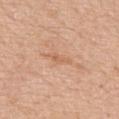{"biopsy_status": "not biopsied; imaged during a skin examination", "patient": {"sex": "male", "age_approx": 75}, "lesion_size": {"long_diameter_mm_approx": 2.5}, "lighting": "white-light", "image": {"source": "total-body photography crop", "field_of_view_mm": 15}, "site": "upper back", "automated_metrics": {"cielab_L": 62, "cielab_a": 22, "cielab_b": 36, "vs_skin_darker_L": 7.0, "vs_skin_contrast_norm": 5.0, "color_variation_0_10": 0.0, "nevus_likeness_0_100": 0, "lesion_detection_confidence_0_100": 100}}